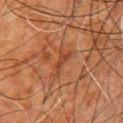workup: total-body-photography surveillance lesion; no biopsy
image source: ~15 mm tile from a whole-body skin photo
size: ≈3.5 mm
location: the chest
subject: male, aged 78–82
tile lighting: cross-polarized illumination